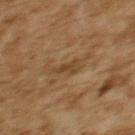The lesion was tiled from a total-body skin photograph and was not biopsied. The recorded lesion diameter is about 3.5 mm. This is a cross-polarized tile. The lesion is on the upper back. A close-up tile cropped from a whole-body skin photograph, about 15 mm across. A female subject aged 58 to 62.